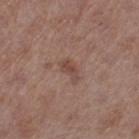No biopsy was performed on this lesion — it was imaged during a full skin examination and was not determined to be concerning. The lesion is on the left thigh. The patient is a male aged around 70. Captured under white-light illumination. Longest diameter approximately 2.5 mm. A roughly 15 mm field-of-view crop from a total-body skin photograph.